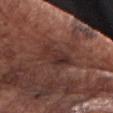Impression: This lesion was catalogued during total-body skin photography and was not selected for biopsy. Acquisition and patient details: From the chest. Imaged with white-light lighting. Automated image analysis of the tile measured an automated nevus-likeness rating near 0 out of 100 and lesion-presence confidence of about 90/100. A male subject, aged 73–77. A 15 mm close-up extracted from a 3D total-body photography capture.